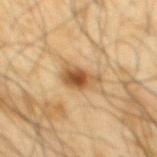Recorded during total-body skin imaging; not selected for excision or biopsy.
The patient is a male aged 63 to 67.
Cropped from a total-body skin-imaging series; the visible field is about 15 mm.
The lesion is located on the mid back.